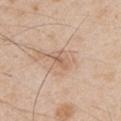Acquisition and patient details: The lesion-visualizer software estimated a mean CIELAB color near L≈62 a*≈18 b*≈30, roughly 8 lightness units darker than nearby skin, and a normalized lesion–skin contrast near 5. Imaged with white-light lighting. Located on the right upper arm. A male patient, aged 48–52. Cropped from a whole-body photographic skin survey; the tile spans about 15 mm.